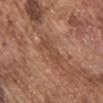The lesion was photographed on a routine skin check and not biopsied; there is no pathology result. Located on the chest. The tile uses white-light illumination. Cropped from a whole-body photographic skin survey; the tile spans about 15 mm. The lesion's longest dimension is about 3.5 mm. A male subject, aged approximately 75.